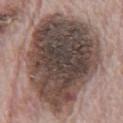Impression:
The lesion was tiled from a total-body skin photograph and was not biopsied.
Image and clinical context:
Imaged with white-light lighting. A male subject, approximately 70 years of age. Automated image analysis of the tile measured an eccentricity of roughly 0.7 and a shape-asymmetry score of about 0.2 (0 = symmetric). And it measured border irregularity of about 3 on a 0–10 scale, a within-lesion color-variation index near 8/10, and radial color variation of about 2.5. The lesion's longest dimension is about 13.5 mm. A roughly 15 mm field-of-view crop from a total-body skin photograph. On the abdomen.